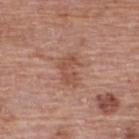• follow-up: imaged on a skin check; not biopsied
• tile lighting: white-light illumination
• subject: female, aged 58 to 62
• image source: total-body-photography crop, ~15 mm field of view
• lesion diameter: ~4 mm (longest diameter)
• location: the back